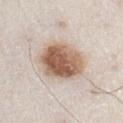Notes:
– site · the abdomen
– lesion size · about 6 mm
– image · ~15 mm crop, total-body skin-cancer survey
– patient · male, about 80 years old
– tile lighting · white-light illumination